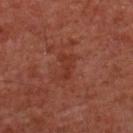workup = imaged on a skin check; not biopsied | subject = male, aged 58 to 62 | TBP lesion metrics = a border-irregularity rating of about 3.5/10 and a color-variation rating of about 1/10; an automated nevus-likeness rating near 0 out of 100 and a detector confidence of about 100 out of 100 that the crop contains a lesion | image = ~15 mm tile from a whole-body skin photo | location = the front of the torso | lighting = cross-polarized illumination | size = ≈3 mm.